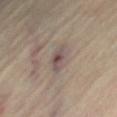Findings:
* follow-up — catalogued during a skin exam; not biopsied
* imaging modality — total-body-photography crop, ~15 mm field of view
* patient — female, aged around 65
* automated metrics — a lesion color around L≈51 a*≈13 b*≈19 in CIELAB, about 9 CIELAB-L* units darker than the surrounding skin, and a normalized border contrast of about 7.5; a border-irregularity rating of about 2.5/10, a within-lesion color-variation index near 5.5/10, and peripheral color asymmetry of about 1.5
* location — the right thigh